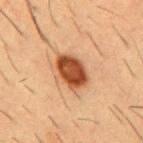A male subject, in their mid- to late 50s.
On the chest.
Automated image analysis of the tile measured a border-irregularity rating of about 1.5/10 and a color-variation rating of about 4/10.
A lesion tile, about 15 mm wide, cut from a 3D total-body photograph.
Imaged with cross-polarized lighting.
The recorded lesion diameter is about 4.5 mm.